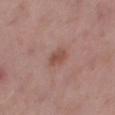The lesion was photographed on a routine skin check and not biopsied; there is no pathology result. The lesion's longest dimension is about 3 mm. The lesion is on the right thigh. Cropped from a total-body skin-imaging series; the visible field is about 15 mm. Captured under white-light illumination. The lesion-visualizer software estimated a lesion area of about 4 mm². A male patient in their mid- to late 50s.